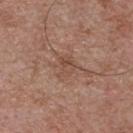Assessment:
The lesion was photographed on a routine skin check and not biopsied; there is no pathology result.
Context:
The subject is a male roughly 55 years of age. Imaged with white-light lighting. Automated tile analysis of the lesion measured a mean CIELAB color near L≈48 a*≈19 b*≈27. And it measured border irregularity of about 4 on a 0–10 scale, a within-lesion color-variation index near 4.5/10, and radial color variation of about 2. A lesion tile, about 15 mm wide, cut from a 3D total-body photograph. The lesion is located on the chest.